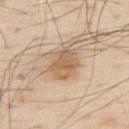The lesion-visualizer software estimated a border-irregularity rating of about 2/10, a within-lesion color-variation index near 4/10, and radial color variation of about 1.5. The tile uses white-light illumination. A male subject in their 80s. The lesion's longest dimension is about 4 mm. Located on the upper back. A region of skin cropped from a whole-body photographic capture, roughly 15 mm wide.